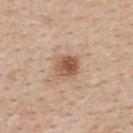Case summary:
* notes: total-body-photography surveillance lesion; no biopsy
* lesion diameter: ~3 mm (longest diameter)
* location: the upper back
* patient: female, in their mid-40s
* automated metrics: an area of roughly 7 mm² and a symmetry-axis asymmetry near 0.2; a border-irregularity rating of about 2/10, a within-lesion color-variation index near 6/10, and radial color variation of about 2
* acquisition: ~15 mm tile from a whole-body skin photo